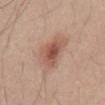site=the front of the torso
diameter=~4.5 mm (longest diameter)
image source=~15 mm tile from a whole-body skin photo
automated lesion analysis=an eccentricity of roughly 0.7; a nevus-likeness score of about 85/100 and a detector confidence of about 100 out of 100 that the crop contains a lesion
lighting=white-light
subject=male, about 30 years old